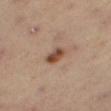A 15 mm close-up extracted from a 3D total-body photography capture.
A male patient aged 53 to 57.
Imaged with cross-polarized lighting.
The recorded lesion diameter is about 3 mm.
Located on the leg.